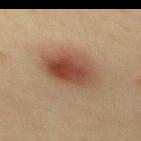Q: Is there a histopathology result?
A: total-body-photography surveillance lesion; no biopsy
Q: What is the anatomic site?
A: the mid back
Q: What are the patient's age and sex?
A: male, approximately 40 years of age
Q: How was this image acquired?
A: ~15 mm tile from a whole-body skin photo
Q: What is the lesion's diameter?
A: ≈5.5 mm